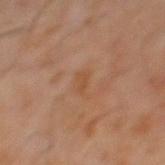workup: imaged on a skin check; not biopsied | body site: the mid back | patient: male, approximately 60 years of age | imaging modality: 15 mm crop, total-body photography | automated metrics: a footprint of about 3 mm², a shape eccentricity near 0.85, and a symmetry-axis asymmetry near 0.25; a lesion color around L≈46 a*≈20 b*≈32 in CIELAB and a normalized lesion–skin contrast near 5; a border-irregularity index near 2.5/10 and a peripheral color-asymmetry measure near 0.5; a classifier nevus-likeness of about 0/100 and a detector confidence of about 100 out of 100 that the crop contains a lesion | illumination: cross-polarized illumination.